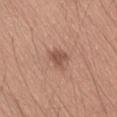  biopsy_status: not biopsied; imaged during a skin examination
  patient:
    sex: male
    age_approx: 55
  lesion_size:
    long_diameter_mm_approx: 3.0
  site: lower back
  lighting: white-light
  automated_metrics:
    eccentricity: 0.65
    shape_asymmetry: 0.3
    cielab_L: 52
    cielab_a: 21
    cielab_b: 27
    vs_skin_darker_L: 10.0
    vs_skin_contrast_norm: 7.0
    nevus_likeness_0_100: 65
    lesion_detection_confidence_0_100: 100
  image:
    source: total-body photography crop
    field_of_view_mm: 15